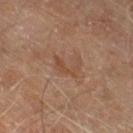Q: Is there a histopathology result?
A: catalogued during a skin exam; not biopsied
Q: What lighting was used for the tile?
A: cross-polarized illumination
Q: What is the anatomic site?
A: the right thigh
Q: What are the patient's age and sex?
A: male, aged 68–72
Q: How was this image acquired?
A: total-body-photography crop, ~15 mm field of view